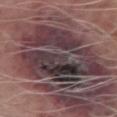{"image": {"source": "total-body photography crop", "field_of_view_mm": 15}, "lesion_size": {"long_diameter_mm_approx": 14.0}, "patient": {"sex": "male", "age_approx": 65}, "site": "left forearm"}